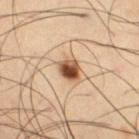This lesion was catalogued during total-body skin photography and was not selected for biopsy.
Cropped from a total-body skin-imaging series; the visible field is about 15 mm.
The lesion-visualizer software estimated roughly 18 lightness units darker than nearby skin. The software also gave a nevus-likeness score of about 100/100 and a lesion-detection confidence of about 100/100.
The patient is a male about 65 years old.
From the right thigh.